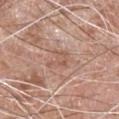The lesion was tiled from a total-body skin photograph and was not biopsied. A region of skin cropped from a whole-body photographic capture, roughly 15 mm wide. A male patient aged around 65. Longest diameter approximately 3 mm. The lesion is located on the chest.